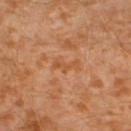Impression: Imaged during a routine full-body skin examination; the lesion was not biopsied and no histopathology is available. Background: Located on the left lower leg. A lesion tile, about 15 mm wide, cut from a 3D total-body photograph. This is a cross-polarized tile. Longest diameter approximately 3.5 mm. The patient is a male approximately 30 years of age.